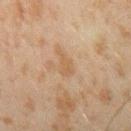Assessment:
Imaged during a routine full-body skin examination; the lesion was not biopsied and no histopathology is available.
Acquisition and patient details:
From the right forearm. Imaged with cross-polarized lighting. A male subject, aged around 45. Measured at roughly 3 mm in maximum diameter. A 15 mm crop from a total-body photograph taken for skin-cancer surveillance.